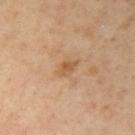<record>
<biopsy_status>not biopsied; imaged during a skin examination</biopsy_status>
<patient>
  <sex>male</sex>
  <age_approx>55</age_approx>
</patient>
<lesion_size>
  <long_diameter_mm_approx>2.5</long_diameter_mm_approx>
</lesion_size>
<image>
  <source>total-body photography crop</source>
  <field_of_view_mm>15</field_of_view_mm>
</image>
<site>arm</site>
<lighting>cross-polarized</lighting>
</record>